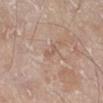Recorded during total-body skin imaging; not selected for excision or biopsy. The lesion's longest dimension is about 2.5 mm. A male patient, roughly 80 years of age. A 15 mm close-up tile from a total-body photography series done for melanoma screening. The lesion is located on the leg.